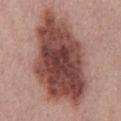| feature | finding |
|---|---|
| notes | total-body-photography surveillance lesion; no biopsy |
| illumination | white-light |
| image | ~15 mm crop, total-body skin-cancer survey |
| location | the chest |
| subject | male, aged approximately 60 |
| automated metrics | a footprint of about 60 mm², an outline eccentricity of about 0.85 (0 = round, 1 = elongated), and a shape-asymmetry score of about 0.25 (0 = symmetric); a mean CIELAB color near L≈46 a*≈23 b*≈23, a lesion–skin lightness drop of about 18, and a normalized border contrast of about 12.5; a color-variation rating of about 7/10 and radial color variation of about 2; a detector confidence of about 100 out of 100 that the crop contains a lesion |
| size | ≈13 mm |